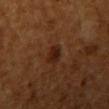Case summary:
- follow-up — imaged on a skin check; not biopsied
- tile lighting — cross-polarized illumination
- lesion size — ~2.5 mm (longest diameter)
- acquisition — ~15 mm crop, total-body skin-cancer survey
- image-analysis metrics — a footprint of about 4 mm², a shape eccentricity near 0.6, and a symmetry-axis asymmetry near 0.2; an average lesion color of about L≈23 a*≈21 b*≈27 (CIELAB), a lesion–skin lightness drop of about 8, and a lesion-to-skin contrast of about 9 (normalized; higher = more distinct); a color-variation rating of about 2.5/10 and radial color variation of about 0.5
- body site — the arm
- subject — female, in their mid- to late 50s